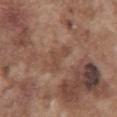biopsy status: no biopsy performed (imaged during a skin exam) | automated metrics: an area of roughly 4.5 mm², an eccentricity of roughly 0.9, and a shape-asymmetry score of about 0.6 (0 = symmetric); a lesion color around L≈46 a*≈19 b*≈27 in CIELAB, roughly 7 lightness units darker than nearby skin, and a normalized lesion–skin contrast near 5.5; an automated nevus-likeness rating near 0 out of 100 and a lesion-detection confidence of about 100/100 | diameter: ≈3.5 mm | imaging modality: ~15 mm tile from a whole-body skin photo | body site: the front of the torso | subject: male, in their mid- to late 70s | illumination: white-light illumination.